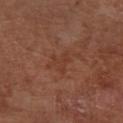Context: Longest diameter approximately 2.5 mm. A lesion tile, about 15 mm wide, cut from a 3D total-body photograph. From the right lower leg. The patient is a male aged around 65. Captured under cross-polarized illumination. Automated tile analysis of the lesion measured a footprint of about 3 mm², a shape eccentricity near 0.4, and a shape-asymmetry score of about 0.7 (0 = symmetric). The software also gave internal color variation of about 0 on a 0–10 scale and peripheral color asymmetry of about 0. The analysis additionally found an automated nevus-likeness rating near 0 out of 100 and a lesion-detection confidence of about 100/100.